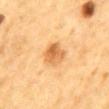This lesion was catalogued during total-body skin photography and was not selected for biopsy. From the abdomen. The tile uses cross-polarized illumination. A male subject, aged 83 or older. This image is a 15 mm lesion crop taken from a total-body photograph. An algorithmic analysis of the crop reported a border-irregularity index near 1.5/10, a within-lesion color-variation index near 5/10, and radial color variation of about 2. The analysis additionally found lesion-presence confidence of about 100/100. About 3.5 mm across.